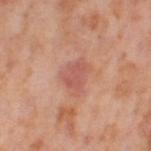Captured during whole-body skin photography for melanoma surveillance; the lesion was not biopsied. A female subject, aged 53–57. From the leg. This image is a 15 mm lesion crop taken from a total-body photograph. The tile uses cross-polarized illumination. An algorithmic analysis of the crop reported an area of roughly 5.5 mm² and an eccentricity of roughly 0.75. The software also gave a lesion color around L≈56 a*≈27 b*≈28 in CIELAB, roughly 8 lightness units darker than nearby skin, and a lesion-to-skin contrast of about 5.5 (normalized; higher = more distinct). And it measured border irregularity of about 3 on a 0–10 scale, internal color variation of about 1.5 on a 0–10 scale, and a peripheral color-asymmetry measure near 0.5. It also reported an automated nevus-likeness rating near 0 out of 100 and a lesion-detection confidence of about 100/100.